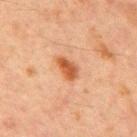Impression:
Recorded during total-body skin imaging; not selected for excision or biopsy.
Image and clinical context:
The lesion is located on the chest. The patient is a male about 65 years old. The lesion's longest dimension is about 3 mm. Captured under cross-polarized illumination. A lesion tile, about 15 mm wide, cut from a 3D total-body photograph.